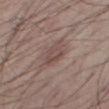Clinical impression: No biopsy was performed on this lesion — it was imaged during a full skin examination and was not determined to be concerning. Background: The lesion is on the right thigh. A male subject aged around 60. A 15 mm crop from a total-body photograph taken for skin-cancer surveillance.